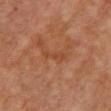Assessment:
Captured during whole-body skin photography for melanoma surveillance; the lesion was not biopsied.
Image and clinical context:
The recorded lesion diameter is about 3.5 mm. A region of skin cropped from a whole-body photographic capture, roughly 15 mm wide. On the chest. The patient is a female aged around 70. This is a cross-polarized tile. Automated image analysis of the tile measured roughly 5 lightness units darker than nearby skin and a normalized border contrast of about 5.5.